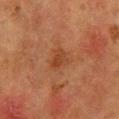Clinical impression: The lesion was tiled from a total-body skin photograph and was not biopsied. Image and clinical context: A close-up tile cropped from a whole-body skin photograph, about 15 mm across. A female patient, about 50 years old. This is a cross-polarized tile. The recorded lesion diameter is about 2.5 mm. Located on the chest.